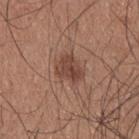{
  "biopsy_status": "not biopsied; imaged during a skin examination",
  "automated_metrics": {
    "cielab_L": 44,
    "cielab_a": 21,
    "cielab_b": 26,
    "vs_skin_darker_L": 10.0,
    "vs_skin_contrast_norm": 7.5,
    "border_irregularity_0_10": 3.5,
    "color_variation_0_10": 3.5,
    "peripheral_color_asymmetry": 1.5,
    "lesion_detection_confidence_0_100": 100
  },
  "lesion_size": {
    "long_diameter_mm_approx": 3.5
  },
  "patient": {
    "sex": "male",
    "age_approx": 25
  },
  "site": "upper back",
  "image": {
    "source": "total-body photography crop",
    "field_of_view_mm": 15
  }
}